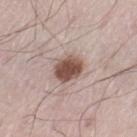| feature | finding |
|---|---|
| anatomic site | the left thigh |
| lesion size | ≈3.5 mm |
| automated metrics | a mean CIELAB color near L≈51 a*≈18 b*≈23, roughly 16 lightness units darker than nearby skin, and a normalized border contrast of about 11; a classifier nevus-likeness of about 95/100 and lesion-presence confidence of about 100/100 |
| patient | male, roughly 55 years of age |
| tile lighting | white-light illumination |
| image | total-body-photography crop, ~15 mm field of view |